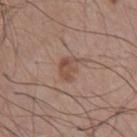Recorded during total-body skin imaging; not selected for excision or biopsy. Longest diameter approximately 3 mm. Automated image analysis of the tile measured a mean CIELAB color near L≈50 a*≈18 b*≈27 and a lesion-to-skin contrast of about 6.5 (normalized; higher = more distinct). A male patient, aged around 75. The tile uses white-light illumination. The lesion is on the chest. A close-up tile cropped from a whole-body skin photograph, about 15 mm across.